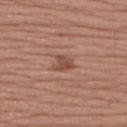- workup · no biopsy performed (imaged during a skin exam)
- diameter · about 2.5 mm
- imaging modality · ~15 mm tile from a whole-body skin photo
- image-analysis metrics · an area of roughly 4 mm², an outline eccentricity of about 0.6 (0 = round, 1 = elongated), and a symmetry-axis asymmetry near 0.35; a lesion–skin lightness drop of about 9
- tile lighting · white-light
- subject · female, approximately 55 years of age
- anatomic site · the upper back